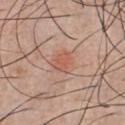No biopsy was performed on this lesion — it was imaged during a full skin examination and was not determined to be concerning. A male subject aged 28 to 32. The lesion is on the chest. This is a white-light tile. Approximately 2.5 mm at its widest. The total-body-photography lesion software estimated a lesion area of about 4 mm², an outline eccentricity of about 0.7 (0 = round, 1 = elongated), and a symmetry-axis asymmetry near 0.25. The analysis additionally found about 7 CIELAB-L* units darker than the surrounding skin and a normalized border contrast of about 5.5. The analysis additionally found internal color variation of about 1.5 on a 0–10 scale. Cropped from a whole-body photographic skin survey; the tile spans about 15 mm.